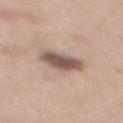  biopsy_status: not biopsied; imaged during a skin examination
  patient:
    sex: female
    age_approx: 40
  automated_metrics:
    nevus_likeness_0_100: 45
    lesion_detection_confidence_0_100: 100
  image:
    source: total-body photography crop
    field_of_view_mm: 15
  lighting: white-light
  lesion_size:
    long_diameter_mm_approx: 5.0
  site: back